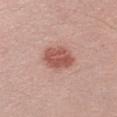Clinical impression: Recorded during total-body skin imaging; not selected for excision or biopsy. Acquisition and patient details: This is a white-light tile. About 4 mm across. A 15 mm close-up tile from a total-body photography series done for melanoma screening. The subject is a male in their mid- to late 20s. From the left upper arm.